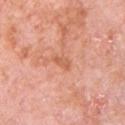Part of a total-body skin-imaging series; this lesion was reviewed on a skin check and was not flagged for biopsy.
The patient is a male roughly 80 years of age.
From the chest.
A close-up tile cropped from a whole-body skin photograph, about 15 mm across.
This is a white-light tile.
An algorithmic analysis of the crop reported an area of roughly 2.5 mm², a shape eccentricity near 0.85, and two-axis asymmetry of about 0.3. The software also gave a lesion color around L≈61 a*≈28 b*≈36 in CIELAB and about 8 CIELAB-L* units darker than the surrounding skin. And it measured a border-irregularity rating of about 3/10 and radial color variation of about 0.5.
Longest diameter approximately 2.5 mm.